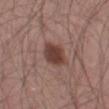This lesion was catalogued during total-body skin photography and was not selected for biopsy. A 15 mm crop from a total-body photograph taken for skin-cancer surveillance. A male subject roughly 55 years of age. Located on the left thigh. Captured under white-light illumination.